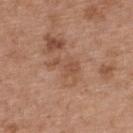Imaged during a routine full-body skin examination; the lesion was not biopsied and no histopathology is available.
A region of skin cropped from a whole-body photographic capture, roughly 15 mm wide.
A female subject roughly 40 years of age.
The lesion is located on the back.